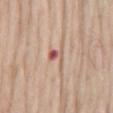<tbp_lesion>
  <biopsy_status>not biopsied; imaged during a skin examination</biopsy_status>
</tbp_lesion>